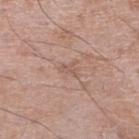The lesion was photographed on a routine skin check and not biopsied; there is no pathology result. Approximately 2.5 mm at its widest. The patient is a male about 60 years old. Located on the right lower leg. This is a white-light tile. A 15 mm close-up tile from a total-body photography series done for melanoma screening.